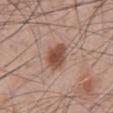Q: What are the patient's age and sex?
A: male, approximately 60 years of age
Q: Where on the body is the lesion?
A: the abdomen
Q: Illumination type?
A: white-light illumination
Q: What is the imaging modality?
A: total-body-photography crop, ~15 mm field of view
Q: Lesion size?
A: ≈3.5 mm
Q: Automated lesion metrics?
A: a lesion color around L≈50 a*≈21 b*≈28 in CIELAB, roughly 13 lightness units darker than nearby skin, and a normalized border contrast of about 9.5; a border-irregularity rating of about 2/10, a color-variation rating of about 4/10, and peripheral color asymmetry of about 1.5